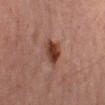Q: Was this lesion biopsied?
A: total-body-photography surveillance lesion; no biopsy
Q: What is the imaging modality?
A: ~15 mm tile from a whole-body skin photo
Q: Who is the patient?
A: female, roughly 70 years of age
Q: Lesion size?
A: ~3.5 mm (longest diameter)
Q: What is the anatomic site?
A: the left thigh
Q: What did automated image analysis measure?
A: an area of roughly 7.5 mm², a shape eccentricity near 0.55, and a shape-asymmetry score of about 0.25 (0 = symmetric); roughly 11 lightness units darker than nearby skin and a lesion-to-skin contrast of about 10 (normalized; higher = more distinct); a border-irregularity index near 2.5/10 and a peripheral color-asymmetry measure near 2
Q: What lighting was used for the tile?
A: cross-polarized